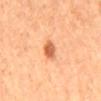{
  "biopsy_status": "not biopsied; imaged during a skin examination",
  "site": "mid back",
  "automated_metrics": {
    "shape_asymmetry": 0.3,
    "border_irregularity_0_10": 3.0
  },
  "patient": {
    "sex": "female",
    "age_approx": 60
  },
  "lesion_size": {
    "long_diameter_mm_approx": 3.5
  },
  "image": {
    "source": "total-body photography crop",
    "field_of_view_mm": 15
  }
}